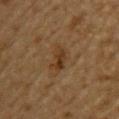Impression:
Recorded during total-body skin imaging; not selected for excision or biopsy.
Background:
A male patient in their mid- to late 80s or older. Located on the upper back. Automated tile analysis of the lesion measured border irregularity of about 4 on a 0–10 scale, a within-lesion color-variation index near 4/10, and radial color variation of about 1.5. The software also gave a classifier nevus-likeness of about 15/100 and lesion-presence confidence of about 100/100. This is a cross-polarized tile. A roughly 15 mm field-of-view crop from a total-body skin photograph. About 3.5 mm across.